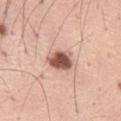Clinical impression:
This lesion was catalogued during total-body skin photography and was not selected for biopsy.
Clinical summary:
A lesion tile, about 15 mm wide, cut from a 3D total-body photograph. On the leg. The patient is a male roughly 60 years of age.